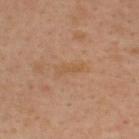Q: Was this lesion biopsied?
A: total-body-photography surveillance lesion; no biopsy
Q: How was the tile lit?
A: cross-polarized
Q: What is the lesion's diameter?
A: ~4 mm (longest diameter)
Q: Lesion location?
A: the upper back
Q: What are the patient's age and sex?
A: male, approximately 35 years of age
Q: Automated lesion metrics?
A: an area of roughly 4.5 mm² and an eccentricity of roughly 0.95; a mean CIELAB color near L≈52 a*≈18 b*≈34 and roughly 5 lightness units darker than nearby skin; border irregularity of about 5 on a 0–10 scale and radial color variation of about 0.5; a nevus-likeness score of about 0/100
Q: How was this image acquired?
A: ~15 mm tile from a whole-body skin photo